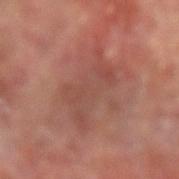This lesion was catalogued during total-body skin photography and was not selected for biopsy. Imaged with cross-polarized lighting. An algorithmic analysis of the crop reported a shape-asymmetry score of about 0.5 (0 = symmetric). The analysis additionally found a lesion color around L≈46 a*≈24 b*≈25 in CIELAB, about 7 CIELAB-L* units darker than the surrounding skin, and a normalized border contrast of about 5. And it measured border irregularity of about 7 on a 0–10 scale, internal color variation of about 3 on a 0–10 scale, and radial color variation of about 1. This image is a 15 mm lesion crop taken from a total-body photograph. On the right forearm. About 7.5 mm across. A male patient aged around 65.